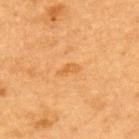| feature | finding |
|---|---|
| follow-up | total-body-photography surveillance lesion; no biopsy |
| location | the upper back |
| image-analysis metrics | a lesion area of about 3 mm², an outline eccentricity of about 0.9 (0 = round, 1 = elongated), and a shape-asymmetry score of about 0.35 (0 = symmetric); a mean CIELAB color near L≈54 a*≈22 b*≈43, a lesion–skin lightness drop of about 6, and a lesion-to-skin contrast of about 5 (normalized; higher = more distinct) |
| lighting | cross-polarized |
| lesion size | about 3 mm |
| subject | female, aged around 40 |
| image | ~15 mm crop, total-body skin-cancer survey |